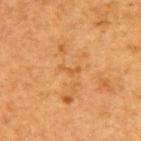The lesion was tiled from a total-body skin photograph and was not biopsied. A 15 mm close-up extracted from a 3D total-body photography capture. On the back. The lesion-visualizer software estimated a lesion color around L≈50 a*≈23 b*≈41 in CIELAB, a lesion–skin lightness drop of about 5, and a normalized lesion–skin contrast near 5. The analysis additionally found a border-irregularity index near 5/10, a color-variation rating of about 0/10, and a peripheral color-asymmetry measure near 0. Captured under cross-polarized illumination. A female subject, roughly 40 years of age. About 2.5 mm across.